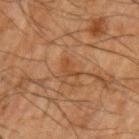Imaged during a routine full-body skin examination; the lesion was not biopsied and no histopathology is available.
A male patient in their mid- to late 60s.
Measured at roughly 2.5 mm in maximum diameter.
Automated tile analysis of the lesion measured a mean CIELAB color near L≈37 a*≈19 b*≈32, roughly 6 lightness units darker than nearby skin, and a normalized border contrast of about 6. And it measured a color-variation rating of about 0/10. The analysis additionally found a classifier nevus-likeness of about 0/100.
Cropped from a whole-body photographic skin survey; the tile spans about 15 mm.
The lesion is located on the left upper arm.